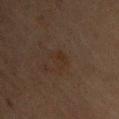| field | value |
|---|---|
| follow-up | imaged on a skin check; not biopsied |
| lesion diameter | about 2.5 mm |
| lighting | cross-polarized |
| automated lesion analysis | an area of roughly 3 mm² and a symmetry-axis asymmetry near 0.55; a classifier nevus-likeness of about 5/100 and a detector confidence of about 100 out of 100 that the crop contains a lesion |
| anatomic site | the chest |
| image | total-body-photography crop, ~15 mm field of view |
| subject | female, aged 63 to 67 |